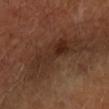No biopsy was performed on this lesion — it was imaged during a full skin examination and was not determined to be concerning. Longest diameter approximately 8.5 mm. A female subject about 60 years old. From the arm. Captured under cross-polarized illumination. A 15 mm crop from a total-body photograph taken for skin-cancer surveillance.